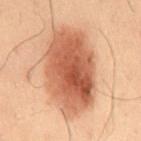Clinical impression:
The lesion was tiled from a total-body skin photograph and was not biopsied.
Context:
A 15 mm close-up tile from a total-body photography series done for melanoma screening. Automated tile analysis of the lesion measured an average lesion color of about L≈47 a*≈22 b*≈29 (CIELAB), about 14 CIELAB-L* units darker than the surrounding skin, and a normalized lesion–skin contrast near 10. It also reported border irregularity of about 2 on a 0–10 scale, a color-variation rating of about 6.5/10, and peripheral color asymmetry of about 2.5. The lesion is on the lower back. A male subject, aged 53–57. The recorded lesion diameter is about 9.5 mm.